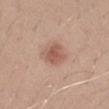Captured under white-light illumination.
The lesion is located on the left forearm.
A male subject, in their 30s.
Cropped from a whole-body photographic skin survey; the tile spans about 15 mm.
Measured at roughly 3 mm in maximum diameter.
Automated tile analysis of the lesion measured a lesion area of about 7.5 mm² and two-axis asymmetry of about 0.2. It also reported a border-irregularity rating of about 2/10. The software also gave an automated nevus-likeness rating near 90 out of 100 and a lesion-detection confidence of about 100/100.